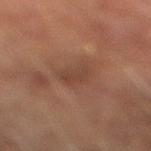Imaged during a routine full-body skin examination; the lesion was not biopsied and no histopathology is available.
A 15 mm crop from a total-body photograph taken for skin-cancer surveillance.
Automated image analysis of the tile measured peripheral color asymmetry of about 0.5. The analysis additionally found a classifier nevus-likeness of about 0/100 and lesion-presence confidence of about 90/100.
A male subject, about 75 years old.
The recorded lesion diameter is about 3 mm.
From the leg.